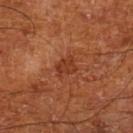Imaged during a routine full-body skin examination; the lesion was not biopsied and no histopathology is available. Imaged with cross-polarized lighting. Automated tile analysis of the lesion measured a lesion area of about 4 mm², a shape eccentricity near 0.6, and two-axis asymmetry of about 0.3. It also reported a border-irregularity rating of about 3/10 and internal color variation of about 2 on a 0–10 scale. It also reported an automated nevus-likeness rating near 5 out of 100 and lesion-presence confidence of about 100/100. The lesion is on the leg. The patient is a male aged around 65. A roughly 15 mm field-of-view crop from a total-body skin photograph. About 2.5 mm across.